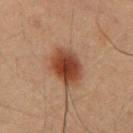Impression: Recorded during total-body skin imaging; not selected for excision or biopsy.